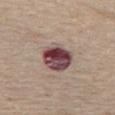Clinical impression:
Recorded during total-body skin imaging; not selected for excision or biopsy.
Image and clinical context:
On the chest. The patient is a female approximately 65 years of age. Automated image analysis of the tile measured a shape eccentricity near 0.7 and two-axis asymmetry of about 0.15. The software also gave a mean CIELAB color near L≈44 a*≈21 b*≈17 and a lesion–skin lightness drop of about 19. And it measured a border-irregularity index near 1.5/10. The analysis additionally found a classifier nevus-likeness of about 5/100 and a lesion-detection confidence of about 100/100. A lesion tile, about 15 mm wide, cut from a 3D total-body photograph. Measured at roughly 5 mm in maximum diameter. The tile uses white-light illumination.